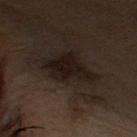biopsy status = no biopsy performed (imaged during a skin exam); location = the head or neck; lighting = cross-polarized; subject = female, aged 58–62; image source = total-body-photography crop, ~15 mm field of view.